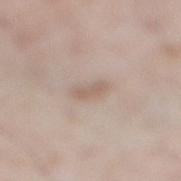{"biopsy_status": "not biopsied; imaged during a skin examination", "automated_metrics": {"area_mm2_approx": 3.5, "shape_asymmetry": 0.3}, "site": "right lower leg", "patient": {"sex": "male", "age_approx": 60}, "lighting": "white-light", "image": {"source": "total-body photography crop", "field_of_view_mm": 15}}